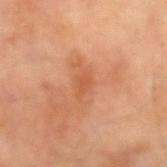The lesion was photographed on a routine skin check and not biopsied; there is no pathology result. Measured at roughly 2.5 mm in maximum diameter. Imaged with cross-polarized lighting. A male patient approximately 70 years of age. An algorithmic analysis of the crop reported a shape eccentricity near 0.8. It also reported a lesion color around L≈55 a*≈27 b*≈38 in CIELAB and a lesion-to-skin contrast of about 5 (normalized; higher = more distinct). It also reported an automated nevus-likeness rating near 0 out of 100. Located on the left forearm. A 15 mm close-up tile from a total-body photography series done for melanoma screening.